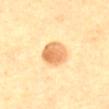Imaged during a routine full-body skin examination; the lesion was not biopsied and no histopathology is available.
Measured at roughly 3.5 mm in maximum diameter.
The subject is a female in their mid- to late 50s.
The lesion is on the abdomen.
The tile uses cross-polarized illumination.
A lesion tile, about 15 mm wide, cut from a 3D total-body photograph.